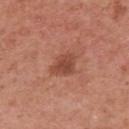No biopsy was performed on this lesion — it was imaged during a full skin examination and was not determined to be concerning. From the right upper arm. A 15 mm close-up extracted from a 3D total-body photography capture. A female subject, in their 50s.